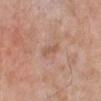notes: imaged on a skin check; not biopsied
lighting: white-light illumination
automated lesion analysis: a footprint of about 3 mm², an eccentricity of roughly 0.95, and a shape-asymmetry score of about 0.2 (0 = symmetric); a mean CIELAB color near L≈56 a*≈21 b*≈30, about 7 CIELAB-L* units darker than the surrounding skin, and a lesion-to-skin contrast of about 5.5 (normalized; higher = more distinct); border irregularity of about 2.5 on a 0–10 scale, a within-lesion color-variation index near 0/10, and a peripheral color-asymmetry measure near 0; an automated nevus-likeness rating near 0 out of 100
location: the left lower leg
imaging modality: ~15 mm crop, total-body skin-cancer survey
subject: male, in their 80s